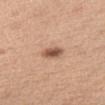Assessment: Imaged during a routine full-body skin examination; the lesion was not biopsied and no histopathology is available. Clinical summary: Approximately 3 mm at its widest. A female subject aged approximately 40. Imaged with white-light lighting. Cropped from a total-body skin-imaging series; the visible field is about 15 mm. Located on the front of the torso. The lesion-visualizer software estimated a lesion color around L≈54 a*≈21 b*≈29 in CIELAB, roughly 14 lightness units darker than nearby skin, and a normalized border contrast of about 9.5. The software also gave a border-irregularity index near 2/10, a color-variation rating of about 3.5/10, and radial color variation of about 1. The analysis additionally found a nevus-likeness score of about 90/100 and a detector confidence of about 100 out of 100 that the crop contains a lesion.